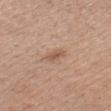<record>
  <biopsy_status>not biopsied; imaged during a skin examination</biopsy_status>
  <lesion_size>
    <long_diameter_mm_approx>2.5</long_diameter_mm_approx>
  </lesion_size>
  <image>
    <source>total-body photography crop</source>
    <field_of_view_mm>15</field_of_view_mm>
  </image>
  <lighting>white-light</lighting>
  <site>head or neck</site>
  <patient>
    <sex>male</sex>
    <age_approx>40</age_approx>
  </patient>
</record>